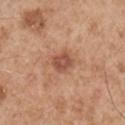Clinical impression:
The lesion was photographed on a routine skin check and not biopsied; there is no pathology result.
Background:
From the right upper arm. Captured under white-light illumination. The patient is a male roughly 55 years of age. A 15 mm crop from a total-body photograph taken for skin-cancer surveillance. Automated image analysis of the tile measured border irregularity of about 2 on a 0–10 scale, a within-lesion color-variation index near 2.5/10, and radial color variation of about 1. It also reported a nevus-likeness score of about 65/100.